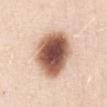biopsy status: total-body-photography surveillance lesion; no biopsy | subject: female, aged 28 to 32 | location: the chest | TBP lesion metrics: border irregularity of about 1.5 on a 0–10 scale and internal color variation of about 8 on a 0–10 scale; an automated nevus-likeness rating near 95 out of 100 and a detector confidence of about 100 out of 100 that the crop contains a lesion | imaging modality: ~15 mm tile from a whole-body skin photo.